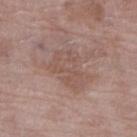Part of a total-body skin-imaging series; this lesion was reviewed on a skin check and was not flagged for biopsy. A female patient, aged approximately 70. The lesion is on the leg. A 15 mm crop from a total-body photograph taken for skin-cancer surveillance. This is a white-light tile. The total-body-photography lesion software estimated a footprint of about 12 mm², an outline eccentricity of about 0.75 (0 = round, 1 = elongated), and a symmetry-axis asymmetry near 0.4. It also reported a border-irregularity index near 5/10, a color-variation rating of about 2.5/10, and peripheral color asymmetry of about 1. And it measured an automated nevus-likeness rating near 0 out of 100.